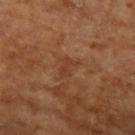A region of skin cropped from a whole-body photographic capture, roughly 15 mm wide. The patient is a male aged 53–57. The recorded lesion diameter is about 3 mm. From the left upper arm. Captured under cross-polarized illumination.